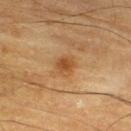Part of a total-body skin-imaging series; this lesion was reviewed on a skin check and was not flagged for biopsy. From the right thigh. The patient is a male aged around 85 or older. A lesion tile, about 15 mm wide, cut from a 3D total-body photograph. Approximately 3 mm at its widest.